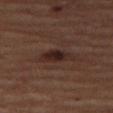notes: no biopsy performed (imaged during a skin exam) | acquisition: ~15 mm tile from a whole-body skin photo | site: the right thigh | subject: female, aged approximately 60 | illumination: cross-polarized | lesion diameter: ~4.5 mm (longest diameter) | TBP lesion metrics: an area of roughly 6.5 mm², a shape eccentricity near 0.9, and a shape-asymmetry score of about 0.3 (0 = symmetric); a detector confidence of about 100 out of 100 that the crop contains a lesion.